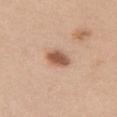{
  "biopsy_status": "not biopsied; imaged during a skin examination",
  "image": {
    "source": "total-body photography crop",
    "field_of_view_mm": 15
  },
  "site": "chest",
  "lighting": "white-light",
  "patient": {
    "sex": "female",
    "age_approx": 40
  },
  "automated_metrics": {
    "eccentricity": 0.75,
    "shape_asymmetry": 0.15,
    "cielab_L": 56,
    "cielab_a": 22,
    "cielab_b": 32,
    "vs_skin_darker_L": 14.0,
    "nevus_likeness_0_100": 100,
    "lesion_detection_confidence_0_100": 100
  },
  "lesion_size": {
    "long_diameter_mm_approx": 3.5
  }
}